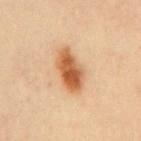{"biopsy_status": "not biopsied; imaged during a skin examination", "lighting": "cross-polarized", "patient": {"sex": "female", "age_approx": 35}, "lesion_size": {"long_diameter_mm_approx": 5.5}, "site": "mid back", "image": {"source": "total-body photography crop", "field_of_view_mm": 15}}